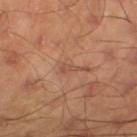Imaged during a routine full-body skin examination; the lesion was not biopsied and no histopathology is available.
A roughly 15 mm field-of-view crop from a total-body skin photograph.
The lesion is located on the right lower leg.
A male subject roughly 45 years of age.